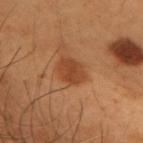Clinical impression:
Part of a total-body skin-imaging series; this lesion was reviewed on a skin check and was not flagged for biopsy.
Image and clinical context:
A male subject aged 53 to 57. Automated tile analysis of the lesion measured an outline eccentricity of about 0.65 (0 = round, 1 = elongated) and a shape-asymmetry score of about 0.2 (0 = symmetric). It also reported an average lesion color of about L≈43 a*≈25 b*≈35 (CIELAB), a lesion–skin lightness drop of about 9, and a lesion-to-skin contrast of about 7.5 (normalized; higher = more distinct). It also reported internal color variation of about 2.5 on a 0–10 scale and peripheral color asymmetry of about 1. The analysis additionally found an automated nevus-likeness rating near 90 out of 100 and a lesion-detection confidence of about 100/100. From the upper back. This image is a 15 mm lesion crop taken from a total-body photograph.